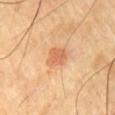Captured during whole-body skin photography for melanoma surveillance; the lesion was not biopsied. The lesion is on the chest. The lesion's longest dimension is about 2.5 mm. Automated tile analysis of the lesion measured a lesion color around L≈61 a*≈25 b*≈37 in CIELAB, roughly 9 lightness units darker than nearby skin, and a normalized border contrast of about 6. The software also gave a border-irregularity rating of about 2.5/10, internal color variation of about 3 on a 0–10 scale, and radial color variation of about 1. And it measured a classifier nevus-likeness of about 45/100 and lesion-presence confidence of about 100/100. This image is a 15 mm lesion crop taken from a total-body photograph. A male patient in their mid- to late 60s.